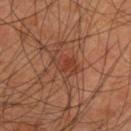This lesion was catalogued during total-body skin photography and was not selected for biopsy.
The patient is a male aged around 45.
This is a cross-polarized tile.
On the upper back.
A roughly 15 mm field-of-view crop from a total-body skin photograph.